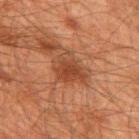A 15 mm crop from a total-body photograph taken for skin-cancer surveillance. Located on the upper back. About 4 mm across. Captured under cross-polarized illumination. A male subject, aged approximately 60. The total-body-photography lesion software estimated roughly 8 lightness units darker than nearby skin. The analysis additionally found a border-irregularity rating of about 4/10, a within-lesion color-variation index near 3/10, and a peripheral color-asymmetry measure near 1.